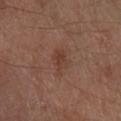biopsy status = total-body-photography surveillance lesion; no biopsy
illumination = cross-polarized illumination
automated metrics = a lesion area of about 4.5 mm² and a shape eccentricity near 0.7; an average lesion color of about L≈37 a*≈20 b*≈25 (CIELAB) and a normalized lesion–skin contrast near 5.5; border irregularity of about 3.5 on a 0–10 scale, a color-variation rating of about 2.5/10, and peripheral color asymmetry of about 1; an automated nevus-likeness rating near 5 out of 100 and lesion-presence confidence of about 100/100
size = ≈3 mm
image source = ~15 mm crop, total-body skin-cancer survey
subject = male, aged 68–72
site = the right lower leg